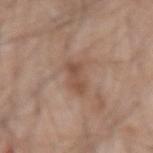Case summary:
– notes — total-body-photography surveillance lesion; no biopsy
– anatomic site — the left forearm
– automated metrics — a lesion area of about 5 mm² and a shape-asymmetry score of about 0.3 (0 = symmetric); a border-irregularity index near 3.5/10, a color-variation rating of about 3.5/10, and peripheral color asymmetry of about 1; a nevus-likeness score of about 0/100 and a lesion-detection confidence of about 100/100
– subject — male, aged 43–47
– imaging modality — ~15 mm tile from a whole-body skin photo
– tile lighting — white-light